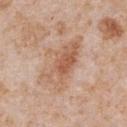| field | value |
|---|---|
| lesion size | ~7 mm (longest diameter) |
| automated lesion analysis | roughly 9 lightness units darker than nearby skin and a normalized border contrast of about 6.5 |
| subject | male, approximately 65 years of age |
| acquisition | ~15 mm tile from a whole-body skin photo |
| body site | the chest |
| lighting | white-light |